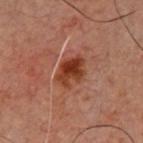notes — no biopsy performed (imaged during a skin exam) | image source — total-body-photography crop, ~15 mm field of view | patient — male, roughly 60 years of age | TBP lesion metrics — a lesion color around L≈29 a*≈22 b*≈25 in CIELAB, a lesion–skin lightness drop of about 9, and a normalized lesion–skin contrast near 10; a border-irregularity index near 2.5/10, internal color variation of about 4.5 on a 0–10 scale, and peripheral color asymmetry of about 1.5; a classifier nevus-likeness of about 90/100 and lesion-presence confidence of about 100/100 | site — the chest | lesion size — about 4 mm.